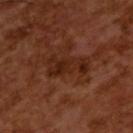Impression: The lesion was photographed on a routine skin check and not biopsied; there is no pathology result. Background: The lesion-visualizer software estimated a classifier nevus-likeness of about 0/100 and a lesion-detection confidence of about 100/100. A male patient, aged around 65. Cropped from a total-body skin-imaging series; the visible field is about 15 mm. The tile uses cross-polarized illumination.